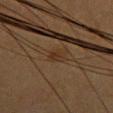Q: Was this lesion biopsied?
A: imaged on a skin check; not biopsied
Q: Who is the patient?
A: female, approximately 55 years of age
Q: What kind of image is this?
A: ~15 mm tile from a whole-body skin photo
Q: Lesion location?
A: the chest